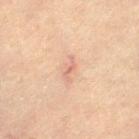Assessment:
The lesion was photographed on a routine skin check and not biopsied; there is no pathology result.
Image and clinical context:
Automated tile analysis of the lesion measured a border-irregularity rating of about 7/10, a color-variation rating of about 0/10, and radial color variation of about 0. The lesion is on the leg. A 15 mm close-up tile from a total-body photography series done for melanoma screening. The patient is a female in their mid- to late 60s. Measured at roughly 3 mm in maximum diameter.